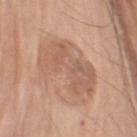About 7.5 mm across.
The tile uses white-light illumination.
The lesion is located on the left upper arm.
A region of skin cropped from a whole-body photographic capture, roughly 15 mm wide.
The subject is a male about 80 years old.